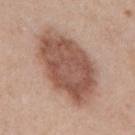Notes:
– follow-up: total-body-photography surveillance lesion; no biopsy
– site: the mid back
– imaging modality: ~15 mm crop, total-body skin-cancer survey
– automated lesion analysis: a lesion area of about 38 mm², a shape eccentricity near 0.85, and two-axis asymmetry of about 0.15; an average lesion color of about L≈53 a*≈21 b*≈27 (CIELAB) and about 14 CIELAB-L* units darker than the surrounding skin
– patient: female, aged 38–42
– lesion size: about 10.5 mm
– lighting: white-light